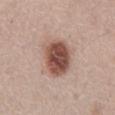workup = no biopsy performed (imaged during a skin exam); site = the abdomen; tile lighting = white-light illumination; patient = male, approximately 65 years of age; imaging modality = ~15 mm crop, total-body skin-cancer survey; diameter = ≈5 mm.